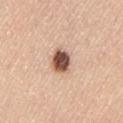{"automated_metrics": {"eccentricity": 0.65, "shape_asymmetry": 0.2, "cielab_L": 54, "cielab_a": 20, "cielab_b": 28, "vs_skin_contrast_norm": 12.5, "border_irregularity_0_10": 1.5, "color_variation_0_10": 7.0, "peripheral_color_asymmetry": 2.0}, "site": "lower back", "patient": {"sex": "female", "age_approx": 65}, "image": {"source": "total-body photography crop", "field_of_view_mm": 15}}